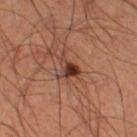| key | value |
|---|---|
| workup | catalogued during a skin exam; not biopsied |
| patient | male, about 50 years old |
| tile lighting | cross-polarized |
| imaging modality | ~15 mm crop, total-body skin-cancer survey |
| location | the right thigh |